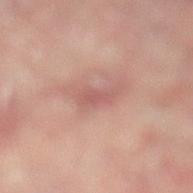Imaged during a routine full-body skin examination; the lesion was not biopsied and no histopathology is available. The lesion-visualizer software estimated an outline eccentricity of about 0.85 (0 = round, 1 = elongated) and two-axis asymmetry of about 0.4. The analysis additionally found border irregularity of about 4.5 on a 0–10 scale, internal color variation of about 1 on a 0–10 scale, and radial color variation of about 0.5. The subject is a male in their 60s. Captured under cross-polarized illumination. The lesion is located on the leg. The recorded lesion diameter is about 3 mm. A lesion tile, about 15 mm wide, cut from a 3D total-body photograph.